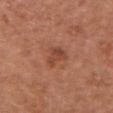Notes:
* workup · imaged on a skin check; not biopsied